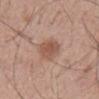The tile uses white-light illumination. A male patient, roughly 55 years of age. Cropped from a whole-body photographic skin survey; the tile spans about 15 mm. The total-body-photography lesion software estimated roughly 9 lightness units darker than nearby skin. The analysis additionally found a border-irregularity rating of about 2.5/10. The software also gave an automated nevus-likeness rating near 90 out of 100 and a detector confidence of about 100 out of 100 that the crop contains a lesion. The lesion is on the mid back.